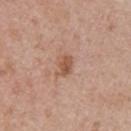| key | value |
|---|---|
| workup | total-body-photography surveillance lesion; no biopsy |
| site | the left upper arm |
| lighting | white-light |
| automated metrics | border irregularity of about 2 on a 0–10 scale and peripheral color asymmetry of about 1 |
| subject | female, aged 58–62 |
| imaging modality | ~15 mm tile from a whole-body skin photo |
| size | ~3 mm (longest diameter) |